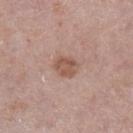Assessment:
Recorded during total-body skin imaging; not selected for excision or biopsy.
Context:
A male patient aged 73–77. The lesion-visualizer software estimated an area of roughly 5.5 mm² and a shape-asymmetry score of about 0.2 (0 = symmetric). It also reported a within-lesion color-variation index near 2.5/10 and a peripheral color-asymmetry measure near 1. This is a white-light tile. Longest diameter approximately 3 mm. A roughly 15 mm field-of-view crop from a total-body skin photograph. On the left lower leg.